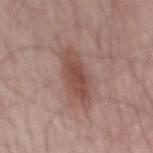Q: Was this lesion biopsied?
A: imaged on a skin check; not biopsied
Q: What kind of image is this?
A: ~15 mm tile from a whole-body skin photo
Q: What did automated image analysis measure?
A: a border-irregularity rating of about 2.5/10, internal color variation of about 3.5 on a 0–10 scale, and a peripheral color-asymmetry measure near 1
Q: Lesion location?
A: the mid back
Q: Who is the patient?
A: male, in their mid- to late 60s
Q: Illumination type?
A: white-light illumination